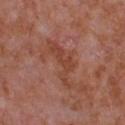A roughly 15 mm field-of-view crop from a total-body skin photograph. The total-body-photography lesion software estimated a lesion color around L≈44 a*≈25 b*≈30 in CIELAB and about 7 CIELAB-L* units darker than the surrounding skin. And it measured a classifier nevus-likeness of about 0/100. A male patient in their mid- to late 60s. The tile uses white-light illumination. The recorded lesion diameter is about 6.5 mm. The lesion is on the front of the torso.